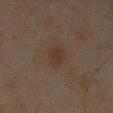<lesion>
<biopsy_status>not biopsied; imaged during a skin examination</biopsy_status>
<patient>
  <sex>male</sex>
  <age_approx>45</age_approx>
</patient>
<image>
  <source>total-body photography crop</source>
  <field_of_view_mm>15</field_of_view_mm>
</image>
<lighting>cross-polarized</lighting>
<lesion_size>
  <long_diameter_mm_approx>3.0</long_diameter_mm_approx>
</lesion_size>
<site>right forearm</site>
<automated_metrics>
  <area_mm2_approx>5.0</area_mm2_approx>
  <eccentricity>0.55</eccentricity>
  <shape_asymmetry>0.25</shape_asymmetry>
  <cielab_L>26</cielab_L>
  <cielab_a>12</cielab_a>
  <cielab_b>20</cielab_b>
  <vs_skin_darker_L>4.0</vs_skin_darker_L>
  <vs_skin_contrast_norm>5.5</vs_skin_contrast_norm>
  <border_irregularity_0_10>2.0</border_irregularity_0_10>
  <color_variation_0_10>2.0</color_variation_0_10>
  <peripheral_color_asymmetry>0.5</peripheral_color_asymmetry>
  <lesion_detection_confidence_0_100>100</lesion_detection_confidence_0_100>
</automated_metrics>
</lesion>